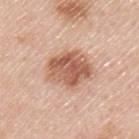Assessment: The lesion was photographed on a routine skin check and not biopsied; there is no pathology result. Acquisition and patient details: A male patient, in their mid-40s. A close-up tile cropped from a whole-body skin photograph, about 15 mm across. The lesion is located on the upper back. The tile uses white-light illumination. Automated image analysis of the tile measured a lesion area of about 17 mm². The analysis additionally found border irregularity of about 2 on a 0–10 scale, a within-lesion color-variation index near 6/10, and radial color variation of about 2.